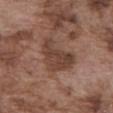follow-up: imaged on a skin check; not biopsied
site: the abdomen
automated metrics: a footprint of about 13 mm², an outline eccentricity of about 0.75 (0 = round, 1 = elongated), and a shape-asymmetry score of about 0.45 (0 = symmetric); a normalized border contrast of about 7.5
tile lighting: white-light
subject: male, about 75 years old
diameter: about 5 mm
acquisition: ~15 mm crop, total-body skin-cancer survey